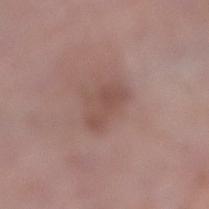follow-up=total-body-photography surveillance lesion; no biopsy | image=~15 mm crop, total-body skin-cancer survey | tile lighting=white-light illumination | image-analysis metrics=an area of roughly 8 mm², a shape eccentricity near 0.75, and two-axis asymmetry of about 0.3; an average lesion color of about L≈50 a*≈19 b*≈23 (CIELAB) and roughly 7 lightness units darker than nearby skin; a border-irregularity index near 3.5/10, a color-variation rating of about 2.5/10, and peripheral color asymmetry of about 1; a nevus-likeness score of about 0/100 and a detector confidence of about 100 out of 100 that the crop contains a lesion | anatomic site=the right lower leg | size=~4 mm (longest diameter) | patient=female, aged 68 to 72.